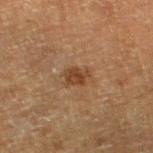The lesion was photographed on a routine skin check and not biopsied; there is no pathology result.